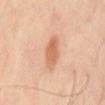No biopsy was performed on this lesion — it was imaged during a full skin examination and was not determined to be concerning. The lesion is located on the mid back. The lesion's longest dimension is about 5 mm. A close-up tile cropped from a whole-body skin photograph, about 15 mm across. Automated tile analysis of the lesion measured a mean CIELAB color near L≈63 a*≈23 b*≈35, roughly 10 lightness units darker than nearby skin, and a lesion-to-skin contrast of about 7 (normalized; higher = more distinct). The software also gave a border-irregularity rating of about 3/10, a within-lesion color-variation index near 3.5/10, and a peripheral color-asymmetry measure near 1.5. It also reported a classifier nevus-likeness of about 90/100 and a lesion-detection confidence of about 100/100. The patient is a male aged around 50.